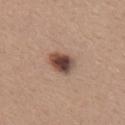Measured at roughly 3.5 mm in maximum diameter. A 15 mm close-up tile from a total-body photography series done for melanoma screening. A female patient, in their 40s. Captured under white-light illumination. From the upper back. The lesion-visualizer software estimated a lesion area of about 6.5 mm², an eccentricity of roughly 0.65, and two-axis asymmetry of about 0.25.